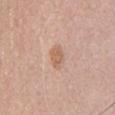Q: How large is the lesion?
A: ≈3 mm
Q: What lighting was used for the tile?
A: white-light illumination
Q: How was this image acquired?
A: total-body-photography crop, ~15 mm field of view
Q: Patient demographics?
A: male, about 60 years old
Q: What is the anatomic site?
A: the chest
Q: What did automated image analysis measure?
A: an area of roughly 4.5 mm², a shape eccentricity near 0.85, and two-axis asymmetry of about 0.3; an average lesion color of about L≈61 a*≈20 b*≈31 (CIELAB); internal color variation of about 2 on a 0–10 scale and a peripheral color-asymmetry measure near 1; a classifier nevus-likeness of about 35/100 and lesion-presence confidence of about 100/100